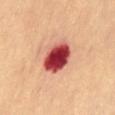Captured under cross-polarized illumination. An algorithmic analysis of the crop reported a footprint of about 12 mm² and an outline eccentricity of about 0.7 (0 = round, 1 = elongated). It also reported an automated nevus-likeness rating near 0 out of 100 and a detector confidence of about 100 out of 100 that the crop contains a lesion. The lesion is on the abdomen. A close-up tile cropped from a whole-body skin photograph, about 15 mm across. The lesion's longest dimension is about 4.5 mm. A female subject, aged 63 to 67.